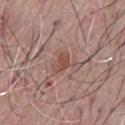lesion diameter: ≈2.5 mm
image: total-body-photography crop, ~15 mm field of view
automated metrics: a footprint of about 3.5 mm², an outline eccentricity of about 0.8 (0 = round, 1 = elongated), and a shape-asymmetry score of about 0.4 (0 = symmetric); a border-irregularity rating of about 4/10, internal color variation of about 1 on a 0–10 scale, and radial color variation of about 0.5
subject: male, in their mid-60s
location: the chest
illumination: white-light illumination